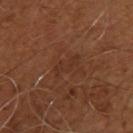| field | value |
|---|---|
| workup | imaged on a skin check; not biopsied |
| tile lighting | cross-polarized illumination |
| site | the upper back |
| patient | male, aged 48 to 52 |
| image source | ~15 mm crop, total-body skin-cancer survey |